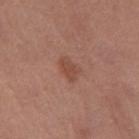follow-up — total-body-photography surveillance lesion; no biopsy
subject — female, approximately 65 years of age
lighting — white-light illumination
diameter — ~3 mm (longest diameter)
image — ~15 mm tile from a whole-body skin photo
body site — the leg